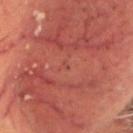Clinical impression: No biopsy was performed on this lesion — it was imaged during a full skin examination and was not determined to be concerning. Clinical summary: About 1 mm across. The total-body-photography lesion software estimated an outline eccentricity of about 0.85 (0 = round, 1 = elongated) and a symmetry-axis asymmetry near 0.45. And it measured a mean CIELAB color near L≈42 a*≈28 b*≈28, about 4 CIELAB-L* units darker than the surrounding skin, and a normalized border contrast of about 3.5. The analysis additionally found a border-irregularity rating of about 3.5/10, a within-lesion color-variation index near 0/10, and radial color variation of about 0. And it measured an automated nevus-likeness rating near 0 out of 100 and a detector confidence of about 100 out of 100 that the crop contains a lesion. Cropped from a whole-body photographic skin survey; the tile spans about 15 mm. Imaged with cross-polarized lighting. A male patient, aged around 60. On the head or neck.